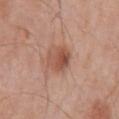This lesion was catalogued during total-body skin photography and was not selected for biopsy.
An algorithmic analysis of the crop reported a footprint of about 7 mm², a shape eccentricity near 0.7, and a shape-asymmetry score of about 0.25 (0 = symmetric). The software also gave a mean CIELAB color near L≈52 a*≈24 b*≈30 and a lesion-to-skin contrast of about 7.5 (normalized; higher = more distinct). It also reported internal color variation of about 5.5 on a 0–10 scale and radial color variation of about 2.
Cropped from a whole-body photographic skin survey; the tile spans about 15 mm.
A male patient aged around 70.
Captured under white-light illumination.
Longest diameter approximately 3.5 mm.
The lesion is on the arm.